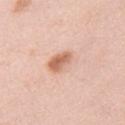Q: Was a biopsy performed?
A: total-body-photography surveillance lesion; no biopsy
Q: Patient demographics?
A: female, aged 38 to 42
Q: What lighting was used for the tile?
A: white-light illumination
Q: What is the anatomic site?
A: the left upper arm
Q: What is the imaging modality?
A: ~15 mm crop, total-body skin-cancer survey